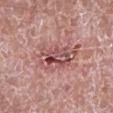{
  "image": {
    "source": "total-body photography crop",
    "field_of_view_mm": 15
  },
  "automated_metrics": {
    "color_variation_0_10": 10.0,
    "nevus_likeness_0_100": 0,
    "lesion_detection_confidence_0_100": 90
  },
  "patient": {
    "sex": "male",
    "age_approx": 65
  },
  "lighting": "white-light",
  "lesion_size": {
    "long_diameter_mm_approx": 6.5
  },
  "site": "leg"
}